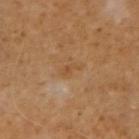{"biopsy_status": "not biopsied; imaged during a skin examination", "image": {"source": "total-body photography crop", "field_of_view_mm": 15}, "patient": {"sex": "male", "age_approx": 55}, "lighting": "cross-polarized", "lesion_size": {"long_diameter_mm_approx": 2.5}, "automated_metrics": {"shape_asymmetry": 0.45, "lesion_detection_confidence_0_100": 100}, "site": "right forearm"}